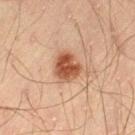Clinical impression: No biopsy was performed on this lesion — it was imaged during a full skin examination and was not determined to be concerning. Background: This image is a 15 mm lesion crop taken from a total-body photograph. The subject is a male in their mid- to late 40s. Located on the left thigh.